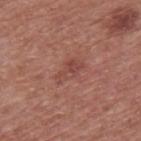Recorded during total-body skin imaging; not selected for excision or biopsy. A male patient aged approximately 75. Captured under white-light illumination. From the back. Measured at roughly 3.5 mm in maximum diameter. A lesion tile, about 15 mm wide, cut from a 3D total-body photograph. An algorithmic analysis of the crop reported a lesion area of about 5.5 mm², a shape eccentricity near 0.85, and two-axis asymmetry of about 0.3. It also reported a border-irregularity rating of about 3.5/10, a color-variation rating of about 3.5/10, and a peripheral color-asymmetry measure near 1. The analysis additionally found an automated nevus-likeness rating near 0 out of 100.